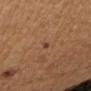notes=total-body-photography surveillance lesion; no biopsy
anatomic site=the right forearm
image source=15 mm crop, total-body photography
patient=female, aged around 35